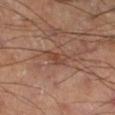Part of a total-body skin-imaging series; this lesion was reviewed on a skin check and was not flagged for biopsy.
Cropped from a whole-body photographic skin survey; the tile spans about 15 mm.
Longest diameter approximately 2.5 mm.
The tile uses cross-polarized illumination.
The lesion is located on the right thigh.